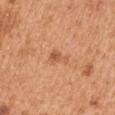<lesion>
  <biopsy_status>not biopsied; imaged during a skin examination</biopsy_status>
  <lesion_size>
    <long_diameter_mm_approx>2.5</long_diameter_mm_approx>
  </lesion_size>
  <image>
    <source>total-body photography crop</source>
    <field_of_view_mm>15</field_of_view_mm>
  </image>
  <site>right upper arm</site>
  <patient>
    <sex>male</sex>
    <age_approx>55</age_approx>
  </patient>
  <automated_metrics>
    <eccentricity>0.8</eccentricity>
    <shape_asymmetry>0.5</shape_asymmetry>
    <border_irregularity_0_10>4.5</border_irregularity_0_10>
    <color_variation_0_10>1.0</color_variation_0_10>
    <peripheral_color_asymmetry>0.5</peripheral_color_asymmetry>
    <nevus_likeness_0_100>10</nevus_likeness_0_100>
    <lesion_detection_confidence_0_100>100</lesion_detection_confidence_0_100>
  </automated_metrics>
</lesion>